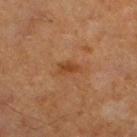biopsy_status: not biopsied; imaged during a skin examination
patient:
  sex: male
  age_approx: 65
site: right lower leg
image:
  source: total-body photography crop
  field_of_view_mm: 15